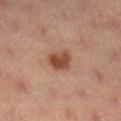Captured during whole-body skin photography for melanoma surveillance; the lesion was not biopsied.
Captured under cross-polarized illumination.
The lesion's longest dimension is about 3 mm.
An algorithmic analysis of the crop reported a lesion color around L≈48 a*≈23 b*≈32 in CIELAB, a lesion–skin lightness drop of about 12, and a normalized border contrast of about 9.5. It also reported border irregularity of about 2 on a 0–10 scale and a within-lesion color-variation index near 4/10.
A lesion tile, about 15 mm wide, cut from a 3D total-body photograph.
A female subject about 35 years old.
The lesion is located on the right lower leg.